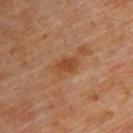{
  "biopsy_status": "not biopsied; imaged during a skin examination",
  "patient": {
    "sex": "male",
    "age_approx": 60
  },
  "lighting": "cross-polarized",
  "site": "back",
  "automated_metrics": {
    "cielab_L": 45,
    "cielab_a": 22,
    "cielab_b": 35,
    "vs_skin_darker_L": 8.0,
    "vs_skin_contrast_norm": 7.0,
    "border_irregularity_0_10": 3.0,
    "color_variation_0_10": 2.0,
    "peripheral_color_asymmetry": 0.5
  },
  "lesion_size": {
    "long_diameter_mm_approx": 3.5
  },
  "image": {
    "source": "total-body photography crop",
    "field_of_view_mm": 15
  }
}